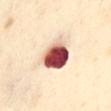<case>
<biopsy_status>not biopsied; imaged during a skin examination</biopsy_status>
<patient>
  <sex>female</sex>
  <age_approx>55</age_approx>
</patient>
<site>front of the torso</site>
<image>
  <source>total-body photography crop</source>
  <field_of_view_mm>15</field_of_view_mm>
</image>
<lesion_size>
  <long_diameter_mm_approx>4.0</long_diameter_mm_approx>
</lesion_size>
</case>